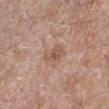Q: Is there a histopathology result?
A: imaged on a skin check; not biopsied
Q: What is the anatomic site?
A: the left lower leg
Q: How was this image acquired?
A: ~15 mm crop, total-body skin-cancer survey
Q: What are the patient's age and sex?
A: male, aged 53 to 57
Q: What lighting was used for the tile?
A: white-light illumination